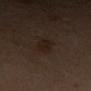The lesion was tiled from a total-body skin photograph and was not biopsied. A female subject, aged 53 to 57. The lesion-visualizer software estimated a mean CIELAB color near L≈19 a*≈12 b*≈19 and a normalized lesion–skin contrast near 7. The lesion is on the left thigh. Captured under cross-polarized illumination. A 15 mm close-up extracted from a 3D total-body photography capture.